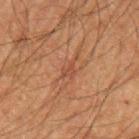<record>
<biopsy_status>not biopsied; imaged during a skin examination</biopsy_status>
<lesion_size>
  <long_diameter_mm_approx>3.0</long_diameter_mm_approx>
</lesion_size>
<site>left upper arm</site>
<image>
  <source>total-body photography crop</source>
  <field_of_view_mm>15</field_of_view_mm>
</image>
<patient>
  <sex>male</sex>
  <age_approx>65</age_approx>
</patient>
</record>